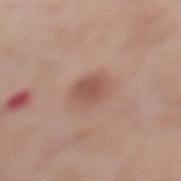Clinical impression: The lesion was photographed on a routine skin check and not biopsied; there is no pathology result. Acquisition and patient details: A lesion tile, about 15 mm wide, cut from a 3D total-body photograph. The lesion is located on the left lower leg. The patient is a female in their mid- to late 50s. Automated tile analysis of the lesion measured an outline eccentricity of about 0.65 (0 = round, 1 = elongated) and two-axis asymmetry of about 0.2. And it measured a mean CIELAB color near L≈53 a*≈22 b*≈26 and roughly 9 lightness units darker than nearby skin. And it measured internal color variation of about 2 on a 0–10 scale and a peripheral color-asymmetry measure near 0.5. And it measured a classifier nevus-likeness of about 95/100 and a detector confidence of about 100 out of 100 that the crop contains a lesion. The tile uses white-light illumination. Longest diameter approximately 3.5 mm.